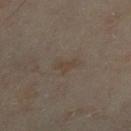Q: Was a biopsy performed?
A: catalogued during a skin exam; not biopsied
Q: How large is the lesion?
A: ~3 mm (longest diameter)
Q: What is the imaging modality?
A: ~15 mm tile from a whole-body skin photo
Q: What are the patient's age and sex?
A: female, in their mid-30s
Q: What lighting was used for the tile?
A: cross-polarized illumination
Q: Lesion location?
A: the right lower leg
Q: Automated lesion metrics?
A: an average lesion color of about L≈38 a*≈10 b*≈22 (CIELAB); a border-irregularity rating of about 4.5/10, internal color variation of about 0.5 on a 0–10 scale, and a peripheral color-asymmetry measure near 0; an automated nevus-likeness rating near 0 out of 100 and a detector confidence of about 100 out of 100 that the crop contains a lesion